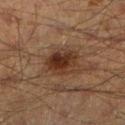Part of a total-body skin-imaging series; this lesion was reviewed on a skin check and was not flagged for biopsy. A close-up tile cropped from a whole-body skin photograph, about 15 mm across. Located on the left lower leg. The tile uses cross-polarized illumination. About 5 mm across. An algorithmic analysis of the crop reported a footprint of about 12 mm², an outline eccentricity of about 0.6 (0 = round, 1 = elongated), and a shape-asymmetry score of about 0.25 (0 = symmetric). It also reported a mean CIELAB color near L≈25 a*≈15 b*≈23 and roughly 8 lightness units darker than nearby skin. And it measured lesion-presence confidence of about 100/100. A male patient, aged 48 to 52.